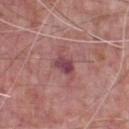Clinical impression: This lesion was catalogued during total-body skin photography and was not selected for biopsy. Image and clinical context: The patient is a male in their 70s. A 15 mm close-up extracted from a 3D total-body photography capture. From the chest.